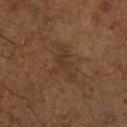Findings:
– biopsy status — imaged on a skin check; not biopsied
– image — ~15 mm tile from a whole-body skin photo
– patient — male, aged 63 to 67
– site — the right lower leg
– lesion diameter — ~5 mm (longest diameter)